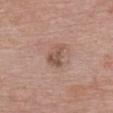The lesion is located on the chest. A roughly 15 mm field-of-view crop from a total-body skin photograph. The subject is a female aged approximately 50.